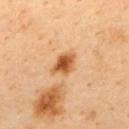Imaged during a routine full-body skin examination; the lesion was not biopsied and no histopathology is available. From the upper back. A 15 mm close-up tile from a total-body photography series done for melanoma screening. A male patient, about 40 years old.